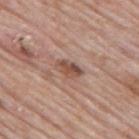Part of a total-body skin-imaging series; this lesion was reviewed on a skin check and was not flagged for biopsy. The lesion is located on the upper back. A lesion tile, about 15 mm wide, cut from a 3D total-body photograph. Captured under white-light illumination. A male patient, in their 60s. The lesion's longest dimension is about 3 mm. Automated tile analysis of the lesion measured a lesion area of about 5 mm², a shape eccentricity near 0.85, and a shape-asymmetry score of about 0.25 (0 = symmetric). The analysis additionally found a lesion color around L≈50 a*≈20 b*≈27 in CIELAB, a lesion–skin lightness drop of about 11, and a normalized lesion–skin contrast near 8. And it measured border irregularity of about 2.5 on a 0–10 scale and a within-lesion color-variation index near 4/10. It also reported a classifier nevus-likeness of about 30/100 and a detector confidence of about 100 out of 100 that the crop contains a lesion.